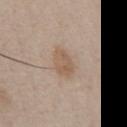Clinical impression: Imaged during a routine full-body skin examination; the lesion was not biopsied and no histopathology is available. Image and clinical context: The recorded lesion diameter is about 3.5 mm. This image is a 15 mm lesion crop taken from a total-body photograph. Imaged with white-light lighting. A male subject approximately 60 years of age. An algorithmic analysis of the crop reported a footprint of about 6.5 mm² and an outline eccentricity of about 0.7 (0 = round, 1 = elongated). The software also gave a mean CIELAB color near L≈57 a*≈15 b*≈29. It also reported internal color variation of about 2.5 on a 0–10 scale and a peripheral color-asymmetry measure near 1. It also reported lesion-presence confidence of about 100/100. Located on the abdomen.